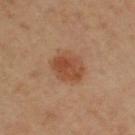workup: no biopsy performed (imaged during a skin exam)
imaging modality: total-body-photography crop, ~15 mm field of view
body site: the arm
size: ≈5 mm
patient: female, about 65 years old